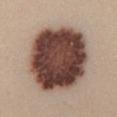This lesion was catalogued during total-body skin photography and was not selected for biopsy. A female patient in their mid- to late 20s. From the mid back. This image is a 15 mm lesion crop taken from a total-body photograph. This is a white-light tile. The lesion's longest dimension is about 8.5 mm.